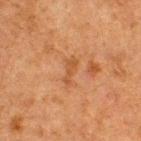workup: total-body-photography surveillance lesion; no biopsy | site: the upper back | subject: male, about 50 years old | image: total-body-photography crop, ~15 mm field of view | illumination: cross-polarized illumination.